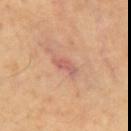The lesion is on the chest.
The subject is a male roughly 65 years of age.
Imaged with cross-polarized lighting.
An algorithmic analysis of the crop reported a within-lesion color-variation index near 1/10 and peripheral color asymmetry of about 0.
A region of skin cropped from a whole-body photographic capture, roughly 15 mm wide.
The lesion's longest dimension is about 3.5 mm.